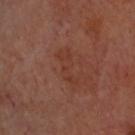Recorded during total-body skin imaging; not selected for excision or biopsy. A close-up tile cropped from a whole-body skin photograph, about 15 mm across. The patient is aged 63–67. The lesion is on the head or neck. Approximately 4.5 mm at its widest.